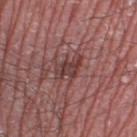Case summary:
* notes: catalogued during a skin exam; not biopsied
* image source: ~15 mm tile from a whole-body skin photo
* anatomic site: the leg
* subject: male, approximately 75 years of age